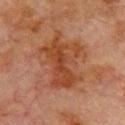{"biopsy_status": "not biopsied; imaged during a skin examination", "lighting": "cross-polarized", "image": {"source": "total-body photography crop", "field_of_view_mm": 15}, "patient": {"sex": "male", "age_approx": 80}, "lesion_size": {"long_diameter_mm_approx": 7.5}, "automated_metrics": {"cielab_L": 34, "cielab_a": 21, "cielab_b": 29, "vs_skin_darker_L": 7.0, "vs_skin_contrast_norm": 8.0, "nevus_likeness_0_100": 0}, "site": "chest"}